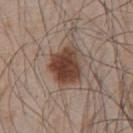Case summary:
* follow-up: total-body-photography surveillance lesion; no biopsy
* image source: ~15 mm crop, total-body skin-cancer survey
* lighting: white-light
* subject: male, about 45 years old
* size: ~4.5 mm (longest diameter)
* site: the chest